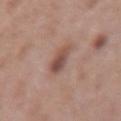<tbp_lesion>
  <biopsy_status>not biopsied; imaged during a skin examination</biopsy_status>
  <site>right forearm</site>
  <image>
    <source>total-body photography crop</source>
    <field_of_view_mm>15</field_of_view_mm>
  </image>
  <patient>
    <sex>female</sex>
    <age_approx>40</age_approx>
  </patient>
</tbp_lesion>